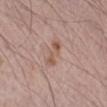| field | value |
|---|---|
| notes | imaged on a skin check; not biopsied |
| subject | male, aged 68 to 72 |
| location | the left forearm |
| acquisition | ~15 mm crop, total-body skin-cancer survey |
| image-analysis metrics | an outline eccentricity of about 0.95 (0 = round, 1 = elongated) and a symmetry-axis asymmetry near 0.3; lesion-presence confidence of about 100/100 |
| tile lighting | white-light illumination |